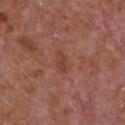Q: Is there a histopathology result?
A: no biopsy performed (imaged during a skin exam)
Q: Who is the patient?
A: male, in their mid- to late 60s
Q: Where on the body is the lesion?
A: the chest
Q: How was this image acquired?
A: total-body-photography crop, ~15 mm field of view